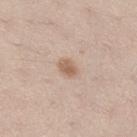The lesion was photographed on a routine skin check and not biopsied; there is no pathology result.
Approximately 2.5 mm at its widest.
The lesion is located on the right thigh.
A lesion tile, about 15 mm wide, cut from a 3D total-body photograph.
A female subject, aged 38 to 42.